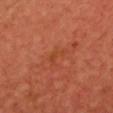Impression:
Part of a total-body skin-imaging series; this lesion was reviewed on a skin check and was not flagged for biopsy.
Image and clinical context:
A 15 mm close-up tile from a total-body photography series done for melanoma screening. Automated tile analysis of the lesion measured a footprint of about 3 mm², a shape eccentricity near 0.9, and a shape-asymmetry score of about 0.65 (0 = symmetric). The software also gave a mean CIELAB color near L≈44 a*≈30 b*≈38 and a lesion–skin lightness drop of about 5. The analysis additionally found internal color variation of about 0 on a 0–10 scale and a peripheral color-asymmetry measure near 0. The analysis additionally found an automated nevus-likeness rating near 0 out of 100 and a lesion-detection confidence of about 95/100. A female patient roughly 50 years of age. About 3 mm across. Captured under cross-polarized illumination.